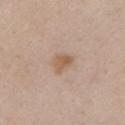Q: Is there a histopathology result?
A: no biopsy performed (imaged during a skin exam)
Q: What is the lesion's diameter?
A: ≈2.5 mm
Q: What is the imaging modality?
A: ~15 mm tile from a whole-body skin photo
Q: What are the patient's age and sex?
A: female, aged 38–42
Q: How was the tile lit?
A: white-light
Q: Lesion location?
A: the chest